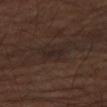tile lighting = cross-polarized illumination; diameter = ~3 mm (longest diameter); site = the right thigh; patient = male, in their 70s; image = ~15 mm tile from a whole-body skin photo.